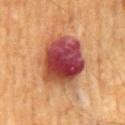This lesion was catalogued during total-body skin photography and was not selected for biopsy. Located on the mid back. The tile uses cross-polarized illumination. A 15 mm close-up tile from a total-body photography series done for melanoma screening. A male subject, aged approximately 70. Automated image analysis of the tile measured a symmetry-axis asymmetry near 0.1. And it measured about 19 CIELAB-L* units darker than the surrounding skin and a normalized lesion–skin contrast near 14.5. It also reported a border-irregularity index near 1.5/10, internal color variation of about 10 on a 0–10 scale, and peripheral color asymmetry of about 4.5. It also reported a classifier nevus-likeness of about 0/100 and a detector confidence of about 100 out of 100 that the crop contains a lesion. The recorded lesion diameter is about 6.5 mm.